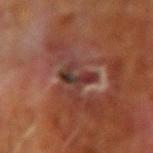illumination — cross-polarized
lesion diameter — ≈4.5 mm
patient — male, about 70 years old
image — 15 mm crop, total-body photography
automated lesion analysis — an area of roughly 5 mm², a shape eccentricity near 0.95, and a symmetry-axis asymmetry near 0.5; border irregularity of about 7.5 on a 0–10 scale, a color-variation rating of about 0.5/10, and a peripheral color-asymmetry measure near 0
location — the right upper arm